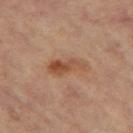patient=female, about 65 years old | illumination=cross-polarized | lesion size=about 4.5 mm | acquisition=~15 mm tile from a whole-body skin photo | site=the right leg.